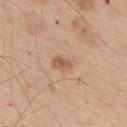Clinical impression:
Imaged during a routine full-body skin examination; the lesion was not biopsied and no histopathology is available.
Image and clinical context:
From the upper back. A male subject, in their mid-50s. A 15 mm close-up tile from a total-body photography series done for melanoma screening. The lesion's longest dimension is about 3.5 mm. This is a white-light tile.